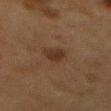biopsy status — no biopsy performed (imaged during a skin exam) | image — ~15 mm tile from a whole-body skin photo | body site — the chest | subject — female, aged 48 to 52 | illumination — cross-polarized.